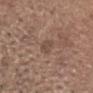notes — imaged on a skin check; not biopsied | image source — ~15 mm crop, total-body skin-cancer survey | anatomic site — the head or neck | subject — male, about 65 years old | automated metrics — an area of roughly 3 mm² and an outline eccentricity of about 0.8 (0 = round, 1 = elongated); a border-irregularity index near 2.5/10, internal color variation of about 1 on a 0–10 scale, and peripheral color asymmetry of about 0; an automated nevus-likeness rating near 0 out of 100 | illumination — white-light.